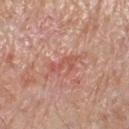{
  "site": "leg",
  "image": {
    "source": "total-body photography crop",
    "field_of_view_mm": 15
  },
  "patient": {
    "sex": "male",
    "age_approx": 80
  }
}